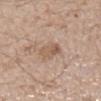image: total-body-photography crop, ~15 mm field of view
automated metrics: an area of roughly 5.5 mm², an outline eccentricity of about 0.65 (0 = round, 1 = elongated), and two-axis asymmetry of about 0.2; a mean CIELAB color near L≈57 a*≈17 b*≈29, about 9 CIELAB-L* units darker than the surrounding skin, and a normalized border contrast of about 6
patient: male, in their mid-60s
body site: the chest
illumination: white-light